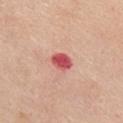Recorded during total-body skin imaging; not selected for excision or biopsy.
The subject is a female roughly 65 years of age.
The recorded lesion diameter is about 2.5 mm.
Captured under white-light illumination.
The lesion is on the front of the torso.
A roughly 15 mm field-of-view crop from a total-body skin photograph.